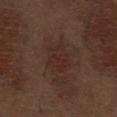workup = total-body-photography surveillance lesion; no biopsy
subject = male, aged 68 to 72
TBP lesion metrics = a lesion area of about 8.5 mm²; an average lesion color of about L≈27 a*≈18 b*≈22 (CIELAB) and roughly 4 lightness units darker than nearby skin; a border-irregularity rating of about 2/10 and a color-variation rating of about 3/10
lighting = white-light illumination
body site = the abdomen
acquisition = total-body-photography crop, ~15 mm field of view